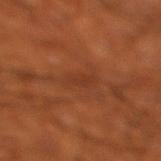Captured during whole-body skin photography for melanoma surveillance; the lesion was not biopsied. From the leg. About 3.5 mm across. The tile uses cross-polarized illumination. Cropped from a whole-body photographic skin survey; the tile spans about 15 mm. Automated tile analysis of the lesion measured an area of roughly 4 mm², an outline eccentricity of about 0.95 (0 = round, 1 = elongated), and two-axis asymmetry of about 0.35. It also reported a border-irregularity index near 4.5/10, internal color variation of about 1 on a 0–10 scale, and a peripheral color-asymmetry measure near 0.5. The analysis additionally found a nevus-likeness score of about 0/100 and a detector confidence of about 50 out of 100 that the crop contains a lesion. A male patient, aged approximately 70.